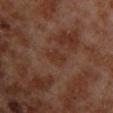Part of a total-body skin-imaging series; this lesion was reviewed on a skin check and was not flagged for biopsy.
A 15 mm close-up tile from a total-body photography series done for melanoma screening.
The lesion is on the left lower leg.
A male subject approximately 70 years of age.